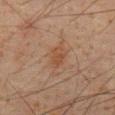Part of a total-body skin-imaging series; this lesion was reviewed on a skin check and was not flagged for biopsy. The patient is a male approximately 60 years of age. On the abdomen. Imaged with cross-polarized lighting. Measured at roughly 2.5 mm in maximum diameter. A lesion tile, about 15 mm wide, cut from a 3D total-body photograph.